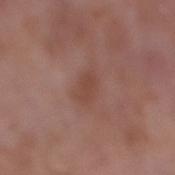Captured during whole-body skin photography for melanoma surveillance; the lesion was not biopsied.
The lesion is located on the right lower leg.
A 15 mm close-up extracted from a 3D total-body photography capture.
Automated tile analysis of the lesion measured an area of roughly 7.5 mm².
Longest diameter approximately 4 mm.
A female subject, in their 70s.